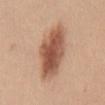biopsy_status: not biopsied; imaged during a skin examination
patient:
  sex: female
  age_approx: 30
image:
  source: total-body photography crop
  field_of_view_mm: 15
site: chest
lesion_size:
  long_diameter_mm_approx: 7.5